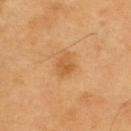follow-up: no biopsy performed (imaged during a skin exam) | anatomic site: the upper back | automated lesion analysis: an average lesion color of about L≈56 a*≈23 b*≈43 (CIELAB), about 8 CIELAB-L* units darker than the surrounding skin, and a normalized lesion–skin contrast near 6; border irregularity of about 2.5 on a 0–10 scale and a color-variation rating of about 2.5/10 | image: total-body-photography crop, ~15 mm field of view | lighting: cross-polarized | patient: male, roughly 60 years of age | diameter: about 2.5 mm.